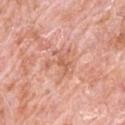Case summary:
– biopsy status · total-body-photography surveillance lesion; no biopsy